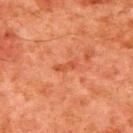The lesion's longest dimension is about 2.5 mm.
A male patient, in their 80s.
A 15 mm crop from a total-body photograph taken for skin-cancer surveillance.
On the upper back.
The tile uses cross-polarized illumination.
The total-body-photography lesion software estimated a lesion color around L≈42 a*≈28 b*≈35 in CIELAB, roughly 6 lightness units darker than nearby skin, and a lesion-to-skin contrast of about 6 (normalized; higher = more distinct). It also reported border irregularity of about 4 on a 0–10 scale, a within-lesion color-variation index near 0/10, and a peripheral color-asymmetry measure near 0. And it measured a nevus-likeness score of about 0/100 and a detector confidence of about 100 out of 100 that the crop contains a lesion.